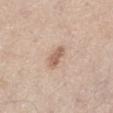Approximately 2.5 mm at its widest.
The patient is a male aged around 75.
A lesion tile, about 15 mm wide, cut from a 3D total-body photograph.
On the left thigh.
An algorithmic analysis of the crop reported a border-irregularity index near 2/10, a color-variation rating of about 2/10, and radial color variation of about 0.5. It also reported an automated nevus-likeness rating near 50 out of 100 and a lesion-detection confidence of about 100/100.